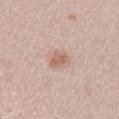  biopsy_status: not biopsied; imaged during a skin examination